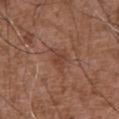Part of a total-body skin-imaging series; this lesion was reviewed on a skin check and was not flagged for biopsy. Captured under white-light illumination. On the abdomen. The lesion's longest dimension is about 3 mm. The subject is a male in their mid-70s. A 15 mm close-up extracted from a 3D total-body photography capture. Automated tile analysis of the lesion measured a border-irregularity rating of about 3.5/10, internal color variation of about 2.5 on a 0–10 scale, and a peripheral color-asymmetry measure near 1.